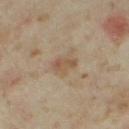Imaged during a routine full-body skin examination; the lesion was not biopsied and no histopathology is available. The subject is a female roughly 35 years of age. The lesion is located on the right thigh. The total-body-photography lesion software estimated an area of roughly 3.5 mm², an eccentricity of roughly 0.85, and a shape-asymmetry score of about 0.3 (0 = symmetric). The analysis additionally found a mean CIELAB color near L≈50 a*≈15 b*≈29, about 8 CIELAB-L* units darker than the surrounding skin, and a lesion-to-skin contrast of about 6.5 (normalized; higher = more distinct). And it measured border irregularity of about 3 on a 0–10 scale, internal color variation of about 2.5 on a 0–10 scale, and radial color variation of about 0.5. The analysis additionally found an automated nevus-likeness rating near 10 out of 100 and lesion-presence confidence of about 100/100. A 15 mm close-up tile from a total-body photography series done for melanoma screening.